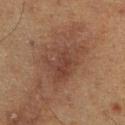workup = catalogued during a skin exam; not biopsied
patient = male, aged approximately 60
lesion size = about 6.5 mm
tile lighting = cross-polarized
location = the right lower leg
automated metrics = an eccentricity of roughly 0.75 and a symmetry-axis asymmetry near 0.35; a color-variation rating of about 4/10 and a peripheral color-asymmetry measure near 1
image source = ~15 mm crop, total-body skin-cancer survey